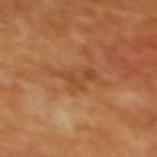{
  "biopsy_status": "not biopsied; imaged during a skin examination"
}